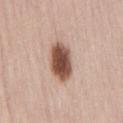The patient is a male aged 43–47. Approximately 5 mm at its widest. The lesion is on the lower back. A 15 mm close-up tile from a total-body photography series done for melanoma screening. Captured under white-light illumination.